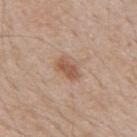Clinical impression: Captured during whole-body skin photography for melanoma surveillance; the lesion was not biopsied. Background: Approximately 3.5 mm at its widest. The lesion is on the mid back. A male patient, aged approximately 50. The lesion-visualizer software estimated a shape-asymmetry score of about 0.3 (0 = symmetric). The software also gave a mean CIELAB color near L≈56 a*≈19 b*≈30 and about 9 CIELAB-L* units darker than the surrounding skin. And it measured border irregularity of about 2.5 on a 0–10 scale and peripheral color asymmetry of about 1. A 15 mm crop from a total-body photograph taken for skin-cancer surveillance. Imaged with white-light lighting.